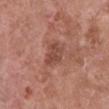biopsy status: total-body-photography surveillance lesion; no biopsy
patient: female, aged approximately 70
lesion diameter: ≈3.5 mm
tile lighting: white-light illumination
location: the front of the torso
acquisition: ~15 mm tile from a whole-body skin photo